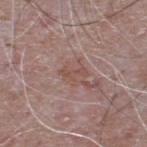follow-up: imaged on a skin check; not biopsied | image source: total-body-photography crop, ~15 mm field of view | body site: the upper back | patient: male, in their mid- to late 60s.